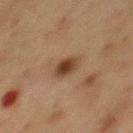Assessment:
This lesion was catalogued during total-body skin photography and was not selected for biopsy.
Clinical summary:
An algorithmic analysis of the crop reported a classifier nevus-likeness of about 100/100 and a lesion-detection confidence of about 100/100. The lesion is located on the front of the torso. The lesion's longest dimension is about 3 mm. A 15 mm close-up extracted from a 3D total-body photography capture. A male patient, roughly 75 years of age.